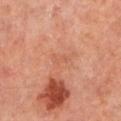Clinical impression: No biopsy was performed on this lesion — it was imaged during a full skin examination and was not determined to be concerning. Image and clinical context: The total-body-photography lesion software estimated a shape-asymmetry score of about 0.5 (0 = symmetric). The analysis additionally found a mean CIELAB color near L≈54 a*≈25 b*≈32, roughly 5 lightness units darker than nearby skin, and a normalized lesion–skin contrast near 3.5. The subject is a male approximately 60 years of age. Cropped from a whole-body photographic skin survey; the tile spans about 15 mm. This is a cross-polarized tile. Located on the left lower leg.